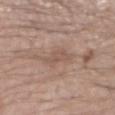{
  "biopsy_status": "not biopsied; imaged during a skin examination",
  "patient": {
    "sex": "male",
    "age_approx": 55
  },
  "image": {
    "source": "total-body photography crop",
    "field_of_view_mm": 15
  },
  "automated_metrics": {
    "area_mm2_approx": 5.5,
    "eccentricity": 0.9,
    "shape_asymmetry": 0.4,
    "cielab_L": 54,
    "cielab_a": 17,
    "cielab_b": 25,
    "vs_skin_darker_L": 7.0,
    "vs_skin_contrast_norm": 5.0,
    "nevus_likeness_0_100": 0,
    "lesion_detection_confidence_0_100": 75
  },
  "site": "left forearm",
  "lesion_size": {
    "long_diameter_mm_approx": 4.5
  }
}